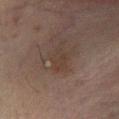follow-up = total-body-photography surveillance lesion; no biopsy
lesion diameter = ~3 mm (longest diameter)
image = ~15 mm crop, total-body skin-cancer survey
illumination = cross-polarized
patient = male, aged approximately 70
location = the left lower leg
image-analysis metrics = a symmetry-axis asymmetry near 0.25; a lesion color around L≈30 a*≈12 b*≈19 in CIELAB and a normalized border contrast of about 4.5